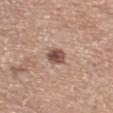This lesion was catalogued during total-body skin photography and was not selected for biopsy.
The recorded lesion diameter is about 2.5 mm.
This is a white-light tile.
From the left lower leg.
Automated image analysis of the tile measured an average lesion color of about L≈50 a*≈19 b*≈25 (CIELAB), about 15 CIELAB-L* units darker than the surrounding skin, and a normalized border contrast of about 10.5. The software also gave border irregularity of about 1.5 on a 0–10 scale, internal color variation of about 3.5 on a 0–10 scale, and radial color variation of about 1.
A lesion tile, about 15 mm wide, cut from a 3D total-body photograph.
A female subject approximately 75 years of age.